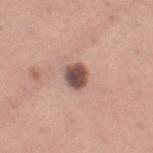Assessment:
Captured during whole-body skin photography for melanoma surveillance; the lesion was not biopsied.
Acquisition and patient details:
A close-up tile cropped from a whole-body skin photograph, about 15 mm across. The tile uses white-light illumination. The lesion is located on the leg. The patient is a female about 30 years old. The lesion's longest dimension is about 3 mm.